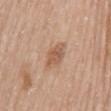Assessment: Imaged during a routine full-body skin examination; the lesion was not biopsied and no histopathology is available. Background: The lesion's longest dimension is about 3.5 mm. A close-up tile cropped from a whole-body skin photograph, about 15 mm across. The tile uses white-light illumination. A female patient in their 70s. On the back.